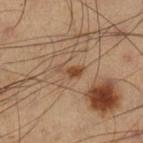No biopsy was performed on this lesion — it was imaged during a full skin examination and was not determined to be concerning. A male subject, about 40 years old. On the leg. A region of skin cropped from a whole-body photographic capture, roughly 15 mm wide.